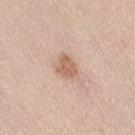Assessment:
Part of a total-body skin-imaging series; this lesion was reviewed on a skin check and was not flagged for biopsy.
Clinical summary:
The lesion's longest dimension is about 3 mm. The lesion is located on the lower back. The tile uses white-light illumination. A female patient, roughly 40 years of age. A lesion tile, about 15 mm wide, cut from a 3D total-body photograph. Automated tile analysis of the lesion measured a nevus-likeness score of about 50/100 and a detector confidence of about 100 out of 100 that the crop contains a lesion.